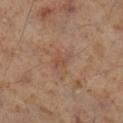{"biopsy_status": "not biopsied; imaged during a skin examination", "patient": {"sex": "male", "age_approx": 60}, "lesion_size": {"long_diameter_mm_approx": 2.5}, "automated_metrics": {"border_irregularity_0_10": 4.0, "color_variation_0_10": 0.5, "nevus_likeness_0_100": 0}, "image": {"source": "total-body photography crop", "field_of_view_mm": 15}, "site": "right lower leg"}